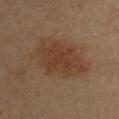Clinical impression: Captured during whole-body skin photography for melanoma surveillance; the lesion was not biopsied. Image and clinical context: Measured at roughly 7 mm in maximum diameter. A 15 mm close-up extracted from a 3D total-body photography capture. From the chest. Automated image analysis of the tile measured an average lesion color of about L≈39 a*≈18 b*≈29 (CIELAB), roughly 8 lightness units darker than nearby skin, and a normalized border contrast of about 7. It also reported border irregularity of about 2.5 on a 0–10 scale, internal color variation of about 2.5 on a 0–10 scale, and peripheral color asymmetry of about 1. The analysis additionally found an automated nevus-likeness rating near 95 out of 100. The patient is a female aged 28–32. Captured under cross-polarized illumination.